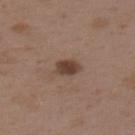Clinical impression: No biopsy was performed on this lesion — it was imaged during a full skin examination and was not determined to be concerning. Clinical summary: This image is a 15 mm lesion crop taken from a total-body photograph. A female patient aged 33 to 37. Located on the upper back.